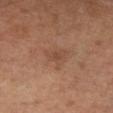biopsy status: imaged on a skin check; not biopsied
automated metrics: a footprint of about 5 mm² and an eccentricity of roughly 0.7; a classifier nevus-likeness of about 5/100 and a detector confidence of about 100 out of 100 that the crop contains a lesion
subject: male, aged 63–67
lesion diameter: ~3 mm (longest diameter)
site: the right lower leg
acquisition: 15 mm crop, total-body photography